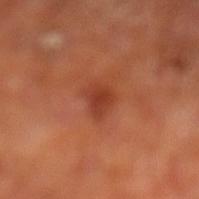Assessment: This lesion was catalogued during total-body skin photography and was not selected for biopsy. Context: Longest diameter approximately 3 mm. A close-up tile cropped from a whole-body skin photograph, about 15 mm across. This is a cross-polarized tile. A male patient about 70 years old. On the left lower leg. Automated tile analysis of the lesion measured a footprint of about 4.5 mm², an eccentricity of roughly 0.7, and a symmetry-axis asymmetry near 0.3. The software also gave a border-irregularity index near 3/10 and a within-lesion color-variation index near 2.5/10. The software also gave a detector confidence of about 100 out of 100 that the crop contains a lesion.